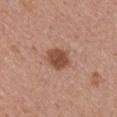Q: Was this lesion biopsied?
A: no biopsy performed (imaged during a skin exam)
Q: Lesion location?
A: the arm
Q: Who is the patient?
A: male, roughly 55 years of age
Q: What is the lesion's diameter?
A: ~3.5 mm (longest diameter)
Q: How was this image acquired?
A: total-body-photography crop, ~15 mm field of view